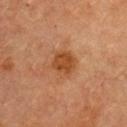This lesion was catalogued during total-body skin photography and was not selected for biopsy.